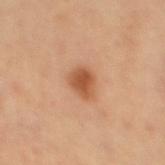A female subject, about 35 years old.
A 15 mm close-up extracted from a 3D total-body photography capture.
Approximately 3 mm at its widest.
Imaged with cross-polarized lighting.
An algorithmic analysis of the crop reported an outline eccentricity of about 0.55 (0 = round, 1 = elongated). It also reported an average lesion color of about L≈54 a*≈26 b*≈38 (CIELAB) and a lesion–skin lightness drop of about 12. And it measured an automated nevus-likeness rating near 100 out of 100 and a lesion-detection confidence of about 100/100.
The lesion is on the right lower leg.